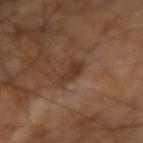{
  "biopsy_status": "not biopsied; imaged during a skin examination",
  "lesion_size": {
    "long_diameter_mm_approx": 3.0
  },
  "automated_metrics": {
    "area_mm2_approx": 4.0,
    "eccentricity": 0.9,
    "shape_asymmetry": 0.25,
    "nevus_likeness_0_100": 0,
    "lesion_detection_confidence_0_100": 100
  },
  "image": {
    "source": "total-body photography crop",
    "field_of_view_mm": 15
  },
  "patient": {
    "age_approx": 65
  },
  "site": "right upper arm"
}